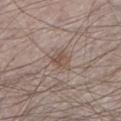notes = total-body-photography surveillance lesion; no biopsy | size = ≈2.5 mm | lighting = white-light | automated lesion analysis = a footprint of about 5 mm² and two-axis asymmetry of about 0.35; a lesion color around L≈51 a*≈15 b*≈24 in CIELAB and about 8 CIELAB-L* units darker than the surrounding skin; an automated nevus-likeness rating near 90 out of 100 and lesion-presence confidence of about 100/100 | anatomic site = the left lower leg | image source = ~15 mm tile from a whole-body skin photo | patient = male, aged approximately 60.